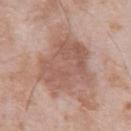The lesion was tiled from a total-body skin photograph and was not biopsied.
A male patient, in their mid-70s.
From the left upper arm.
Captured under white-light illumination.
Longest diameter approximately 7 mm.
This image is a 15 mm lesion crop taken from a total-body photograph.